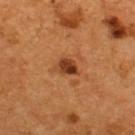Q: Who is the patient?
A: male, aged approximately 55
Q: Lesion size?
A: ≈3 mm
Q: Automated lesion metrics?
A: a footprint of about 5 mm², an outline eccentricity of about 0.65 (0 = round, 1 = elongated), and a shape-asymmetry score of about 0.3 (0 = symmetric); a mean CIELAB color near L≈37 a*≈24 b*≈34, roughly 12 lightness units darker than nearby skin, and a normalized border contrast of about 10; border irregularity of about 2.5 on a 0–10 scale and a peripheral color-asymmetry measure near 1.5; a classifier nevus-likeness of about 80/100 and a lesion-detection confidence of about 100/100
Q: Lesion location?
A: the upper back
Q: What kind of image is this?
A: ~15 mm crop, total-body skin-cancer survey